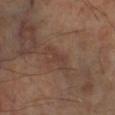Case summary:
- biopsy status · imaged on a skin check; not biopsied